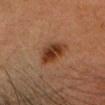- follow-up — total-body-photography surveillance lesion; no biopsy